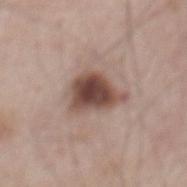Recorded during total-body skin imaging; not selected for excision or biopsy. This is a white-light tile. A male patient, aged approximately 65. An algorithmic analysis of the crop reported an area of roughly 13 mm² and a shape eccentricity near 0.7. The analysis additionally found an average lesion color of about L≈47 a*≈18 b*≈23 (CIELAB), a lesion–skin lightness drop of about 16, and a normalized border contrast of about 11. And it measured an automated nevus-likeness rating near 95 out of 100 and a detector confidence of about 100 out of 100 that the crop contains a lesion. A roughly 15 mm field-of-view crop from a total-body skin photograph. From the mid back.